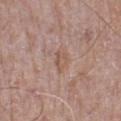  biopsy_status: not biopsied; imaged during a skin examination
  site: left lower leg
  patient:
    sex: male
    age_approx: 80
  lesion_size:
    long_diameter_mm_approx: 2.5
  image:
    source: total-body photography crop
    field_of_view_mm: 15
  lighting: white-light